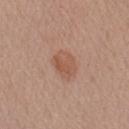{"site": "left upper arm", "patient": {"sex": "male", "age_approx": 60}, "lighting": "white-light", "automated_metrics": {"eccentricity": 0.7, "cielab_L": 54, "cielab_a": 21, "cielab_b": 30, "vs_skin_darker_L": 8.0, "vs_skin_contrast_norm": 6.0}, "lesion_size": {"long_diameter_mm_approx": 3.5}, "image": {"source": "total-body photography crop", "field_of_view_mm": 15}}